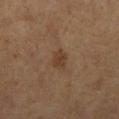notes=catalogued during a skin exam; not biopsied
image source=total-body-photography crop, ~15 mm field of view
illumination=cross-polarized
anatomic site=the right lower leg
lesion diameter=about 2.5 mm
subject=female, aged around 65